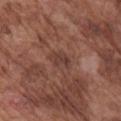Imaged during a routine full-body skin examination; the lesion was not biopsied and no histopathology is available. A close-up tile cropped from a whole-body skin photograph, about 15 mm across. Approximately 3 mm at its widest. A male subject aged around 75. Captured under white-light illumination. The lesion is on the right upper arm. An algorithmic analysis of the crop reported an area of roughly 5 mm², an outline eccentricity of about 0.6 (0 = round, 1 = elongated), and a shape-asymmetry score of about 0.2 (0 = symmetric). It also reported an average lesion color of about L≈40 a*≈21 b*≈24 (CIELAB), a lesion–skin lightness drop of about 7, and a lesion-to-skin contrast of about 6 (normalized; higher = more distinct). The analysis additionally found a classifier nevus-likeness of about 0/100 and lesion-presence confidence of about 100/100.